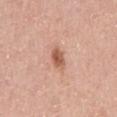{
  "biopsy_status": "not biopsied; imaged during a skin examination",
  "image": {
    "source": "total-body photography crop",
    "field_of_view_mm": 15
  },
  "lesion_size": {
    "long_diameter_mm_approx": 3.0
  },
  "site": "front of the torso",
  "patient": {
    "sex": "male",
    "age_approx": 60
  },
  "lighting": "white-light"
}